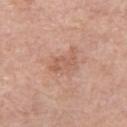Findings:
• follow-up: imaged on a skin check; not biopsied
• subject: female, in their 70s
• body site: the right thigh
• image: total-body-photography crop, ~15 mm field of view